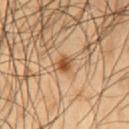Impression:
The lesion was tiled from a total-body skin photograph and was not biopsied.
Image and clinical context:
This is a cross-polarized tile. From the mid back. A male patient roughly 50 years of age. Cropped from a whole-body photographic skin survey; the tile spans about 15 mm. The lesion's longest dimension is about 2.5 mm.